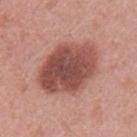Captured during whole-body skin photography for melanoma surveillance; the lesion was not biopsied. Approximately 7.5 mm at its widest. The subject is a female roughly 40 years of age. Located on the left upper arm. A lesion tile, about 15 mm wide, cut from a 3D total-body photograph. Automated image analysis of the tile measured a detector confidence of about 100 out of 100 that the crop contains a lesion.